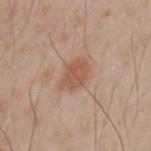Findings:
* notes: catalogued during a skin exam; not biopsied
* subject: male, roughly 50 years of age
* automated lesion analysis: a lesion area of about 7 mm², an eccentricity of roughly 0.7, and a shape-asymmetry score of about 0.15 (0 = symmetric); border irregularity of about 1.5 on a 0–10 scale, a within-lesion color-variation index near 1.5/10, and a peripheral color-asymmetry measure near 0.5; lesion-presence confidence of about 100/100
* lesion size: about 3.5 mm
* anatomic site: the left upper arm
* imaging modality: ~15 mm tile from a whole-body skin photo
* lighting: cross-polarized illumination